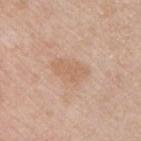On the chest. A male subject aged 43–47. A 15 mm close-up extracted from a 3D total-body photography capture.